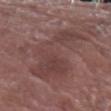  biopsy_status: not biopsied; imaged during a skin examination
  image:
    source: total-body photography crop
    field_of_view_mm: 15
  automated_metrics:
    area_mm2_approx: 29.0
    eccentricity: 0.95
    shape_asymmetry: 0.5
    cielab_L: 41
    cielab_a: 19
    cielab_b: 19
    border_irregularity_0_10: 8.5
    color_variation_0_10: 3.5
    peripheral_color_asymmetry: 1.0
  lesion_size:
    long_diameter_mm_approx: 10.5
  patient:
    sex: female
    age_approx: 70
  site: left forearm